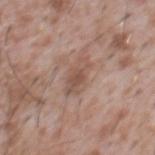Q: Is there a histopathology result?
A: catalogued during a skin exam; not biopsied
Q: Who is the patient?
A: male, approximately 45 years of age
Q: How was the tile lit?
A: white-light illumination
Q: What kind of image is this?
A: ~15 mm tile from a whole-body skin photo
Q: How large is the lesion?
A: ~4.5 mm (longest diameter)
Q: Automated lesion metrics?
A: a mean CIELAB color near L≈52 a*≈18 b*≈26, about 9 CIELAB-L* units darker than the surrounding skin, and a lesion-to-skin contrast of about 6.5 (normalized; higher = more distinct); a border-irregularity index near 3.5/10 and a color-variation rating of about 2.5/10
Q: What is the anatomic site?
A: the front of the torso